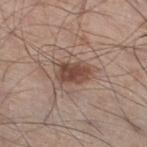notes: catalogued during a skin exam; not biopsied | patient: male, aged 58–62 | body site: the right thigh | image: 15 mm crop, total-body photography | tile lighting: white-light illumination.